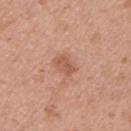This lesion was catalogued during total-body skin photography and was not selected for biopsy. The subject is a female approximately 40 years of age. Captured under white-light illumination. Automated tile analysis of the lesion measured an average lesion color of about L≈56 a*≈24 b*≈31 (CIELAB), roughly 9 lightness units darker than nearby skin, and a normalized border contrast of about 6. The recorded lesion diameter is about 3 mm. From the left upper arm. A 15 mm close-up extracted from a 3D total-body photography capture.